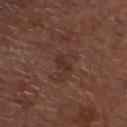Recorded during total-body skin imaging; not selected for excision or biopsy.
The subject is a male aged 58–62.
The lesion is on the head or neck.
Automated image analysis of the tile measured a lesion area of about 2.5 mm², an eccentricity of roughly 0.85, and a symmetry-axis asymmetry near 0.4. The software also gave a classifier nevus-likeness of about 0/100 and a detector confidence of about 100 out of 100 that the crop contains a lesion.
Imaged with cross-polarized lighting.
Cropped from a whole-body photographic skin survey; the tile spans about 15 mm.